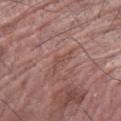Clinical impression: Part of a total-body skin-imaging series; this lesion was reviewed on a skin check and was not flagged for biopsy. Context: This is a white-light tile. A 15 mm close-up tile from a total-body photography series done for melanoma screening. The patient is a male aged 63–67. The lesion's longest dimension is about 3 mm. The lesion is located on the left forearm.